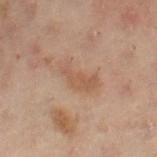biopsy status: no biopsy performed (imaged during a skin exam); image: ~15 mm tile from a whole-body skin photo; body site: the left leg; subject: female, roughly 55 years of age.